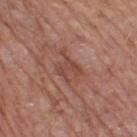Q: Was this lesion biopsied?
A: imaged on a skin check; not biopsied
Q: Lesion location?
A: the right thigh
Q: How was this image acquired?
A: total-body-photography crop, ~15 mm field of view
Q: What lighting was used for the tile?
A: white-light illumination
Q: What are the patient's age and sex?
A: male, roughly 75 years of age
Q: How large is the lesion?
A: ~3 mm (longest diameter)
Q: Automated lesion metrics?
A: a footprint of about 4.5 mm², an eccentricity of roughly 0.75, and a shape-asymmetry score of about 0.45 (0 = symmetric); an average lesion color of about L≈46 a*≈23 b*≈27 (CIELAB), about 7 CIELAB-L* units darker than the surrounding skin, and a lesion-to-skin contrast of about 5.5 (normalized; higher = more distinct); a within-lesion color-variation index near 1.5/10 and a peripheral color-asymmetry measure near 0.5; a lesion-detection confidence of about 100/100